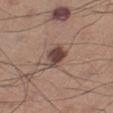| feature | finding |
|---|---|
| notes | total-body-photography surveillance lesion; no biopsy |
| subject | male, aged around 60 |
| TBP lesion metrics | a lesion color around L≈43 a*≈17 b*≈22 in CIELAB, roughly 13 lightness units darker than nearby skin, and a normalized lesion–skin contrast near 10.5; a color-variation rating of about 3/10 and peripheral color asymmetry of about 1; a lesion-detection confidence of about 100/100 |
| anatomic site | the left lower leg |
| lesion diameter | ~3 mm (longest diameter) |
| image source | ~15 mm crop, total-body skin-cancer survey |